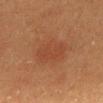Assessment: No biopsy was performed on this lesion — it was imaged during a full skin examination and was not determined to be concerning. Acquisition and patient details: Measured at roughly 4.5 mm in maximum diameter. On the mid back. A female subject about 40 years old. A lesion tile, about 15 mm wide, cut from a 3D total-body photograph.